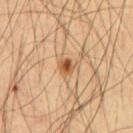Impression:
No biopsy was performed on this lesion — it was imaged during a full skin examination and was not determined to be concerning.
Context:
Measured at roughly 2 mm in maximum diameter. The total-body-photography lesion software estimated an average lesion color of about L≈54 a*≈22 b*≈38 (CIELAB) and a lesion–skin lightness drop of about 13. The software also gave a nevus-likeness score of about 95/100 and a detector confidence of about 100 out of 100 that the crop contains a lesion. A male subject aged 53 to 57. A 15 mm close-up extracted from a 3D total-body photography capture. On the abdomen. Imaged with cross-polarized lighting.